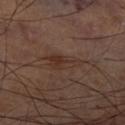The lesion was photographed on a routine skin check and not biopsied; there is no pathology result.
The lesion is located on the right thigh.
The tile uses cross-polarized illumination.
Cropped from a total-body skin-imaging series; the visible field is about 15 mm.